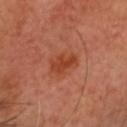Background:
A close-up tile cropped from a whole-body skin photograph, about 15 mm across. The patient is a male approximately 65 years of age. The lesion is located on the head or neck. Captured under cross-polarized illumination. Measured at roughly 3 mm in maximum diameter.